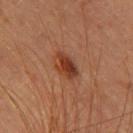Q: Was this lesion biopsied?
A: no biopsy performed (imaged during a skin exam)
Q: Lesion size?
A: about 3 mm
Q: Who is the patient?
A: female, approximately 40 years of age
Q: What kind of image is this?
A: 15 mm crop, total-body photography
Q: How was the tile lit?
A: cross-polarized
Q: Automated lesion metrics?
A: a border-irregularity rating of about 1.5/10 and internal color variation of about 4.5 on a 0–10 scale
Q: Where on the body is the lesion?
A: the back